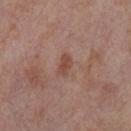- biopsy status · no biopsy performed (imaged during a skin exam)
- image · total-body-photography crop, ~15 mm field of view
- diameter · about 2.5 mm
- TBP lesion metrics · a lesion color around L≈47 a*≈21 b*≈27 in CIELAB, roughly 8 lightness units darker than nearby skin, and a lesion-to-skin contrast of about 7 (normalized; higher = more distinct); a border-irregularity index near 3/10, a color-variation rating of about 1.5/10, and radial color variation of about 0.5
- anatomic site · the leg
- illumination · cross-polarized illumination
- subject · female, in their mid- to late 50s